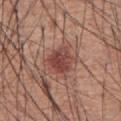This lesion was catalogued during total-body skin photography and was not selected for biopsy. The recorded lesion diameter is about 4 mm. A 15 mm crop from a total-body photograph taken for skin-cancer surveillance. The patient is a male aged approximately 60. This is a white-light tile. The lesion is located on the abdomen.